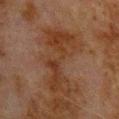Q: Is there a histopathology result?
A: no biopsy performed (imaged during a skin exam)
Q: Patient demographics?
A: male, in their 80s
Q: What is the imaging modality?
A: ~15 mm tile from a whole-body skin photo
Q: Illumination type?
A: cross-polarized
Q: What is the anatomic site?
A: the back